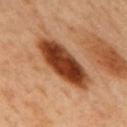Located on the mid back. Imaged with cross-polarized lighting. A close-up tile cropped from a whole-body skin photograph, about 15 mm across. About 8 mm across. A male patient, about 50 years old.